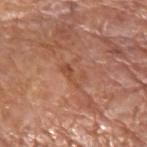The lesion was photographed on a routine skin check and not biopsied; there is no pathology result. A male subject, in their mid-70s. The tile uses white-light illumination. Measured at roughly 3 mm in maximum diameter. On the arm. This image is a 15 mm lesion crop taken from a total-body photograph.